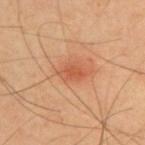{"biopsy_status": "not biopsied; imaged during a skin examination", "patient": {"sex": "male", "age_approx": 65}, "lesion_size": {"long_diameter_mm_approx": 3.0}, "site": "upper back", "lighting": "cross-polarized", "image": {"source": "total-body photography crop", "field_of_view_mm": 15}}